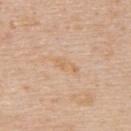notes: catalogued during a skin exam; not biopsied | location: the upper back | acquisition: total-body-photography crop, ~15 mm field of view | subject: male, roughly 60 years of age | tile lighting: white-light | diameter: ≈3.5 mm | image-analysis metrics: a shape eccentricity near 0.9 and two-axis asymmetry of about 0.25; an automated nevus-likeness rating near 0 out of 100 and a detector confidence of about 100 out of 100 that the crop contains a lesion.